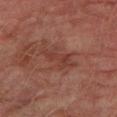Captured during whole-body skin photography for melanoma surveillance; the lesion was not biopsied. A 15 mm crop from a total-body photograph taken for skin-cancer surveillance. On the left lower leg. The subject is a male in their mid-70s. The tile uses cross-polarized illumination. The recorded lesion diameter is about 5.5 mm.